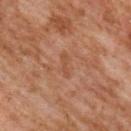Case summary:
* notes — total-body-photography surveillance lesion; no biopsy
* TBP lesion metrics — a shape eccentricity near 0.95; a mean CIELAB color near L≈43 a*≈21 b*≈30 and a lesion–skin lightness drop of about 5; border irregularity of about 4.5 on a 0–10 scale, internal color variation of about 0 on a 0–10 scale, and peripheral color asymmetry of about 0; a classifier nevus-likeness of about 0/100 and a detector confidence of about 100 out of 100 that the crop contains a lesion
* diameter — ~3 mm (longest diameter)
* site — the upper back
* acquisition — ~15 mm tile from a whole-body skin photo
* patient — female, approximately 60 years of age